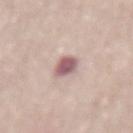Clinical impression: Captured during whole-body skin photography for melanoma surveillance; the lesion was not biopsied. Clinical summary: A close-up tile cropped from a whole-body skin photograph, about 15 mm across. A female subject aged 63 to 67. Located on the lower back.